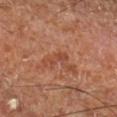notes = catalogued during a skin exam; not biopsied | lighting = cross-polarized | lesion size = about 2.5 mm | image source = ~15 mm crop, total-body skin-cancer survey | automated metrics = a border-irregularity rating of about 4.5/10, a within-lesion color-variation index near 0/10, and radial color variation of about 0 | anatomic site = the right lower leg | subject = aged 63–67.